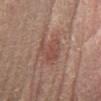| field | value |
|---|---|
| follow-up | no biopsy performed (imaged during a skin exam) |
| site | the left lower leg |
| imaging modality | 15 mm crop, total-body photography |
| lesion size | about 4 mm |
| tile lighting | cross-polarized illumination |
| patient | female, aged around 50 |